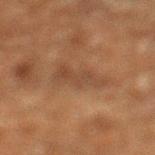{
  "biopsy_status": "not biopsied; imaged during a skin examination",
  "image": {
    "source": "total-body photography crop",
    "field_of_view_mm": 15
  },
  "patient": {
    "sex": "male",
    "age_approx": 60
  },
  "lighting": "cross-polarized",
  "site": "left lower leg",
  "automated_metrics": {
    "area_mm2_approx": 8.5,
    "eccentricity": 0.9,
    "shape_asymmetry": 0.45,
    "nevus_likeness_0_100": 0
  },
  "lesion_size": {
    "long_diameter_mm_approx": 5.5
  }
}